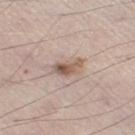Recorded during total-body skin imaging; not selected for excision or biopsy.
A close-up tile cropped from a whole-body skin photograph, about 15 mm across.
From the leg.
A male patient, in their mid- to late 70s.